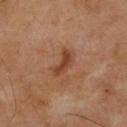From the back.
A male subject roughly 55 years of age.
A 15 mm close-up extracted from a 3D total-body photography capture.
An algorithmic analysis of the crop reported a lesion color around L≈39 a*≈22 b*≈31 in CIELAB, roughly 9 lightness units darker than nearby skin, and a lesion-to-skin contrast of about 8 (normalized; higher = more distinct). The software also gave a border-irregularity index near 4/10, a within-lesion color-variation index near 0.5/10, and a peripheral color-asymmetry measure near 0. It also reported an automated nevus-likeness rating near 0 out of 100 and a detector confidence of about 100 out of 100 that the crop contains a lesion.